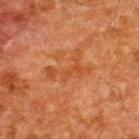Q: Was this lesion biopsied?
A: total-body-photography surveillance lesion; no biopsy
Q: What is the imaging modality?
A: total-body-photography crop, ~15 mm field of view
Q: What are the patient's age and sex?
A: male, approximately 60 years of age
Q: What is the anatomic site?
A: the upper back